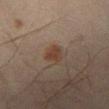biopsy status = no biopsy performed (imaged during a skin exam) | location = the leg | image source = ~15 mm crop, total-body skin-cancer survey | tile lighting = cross-polarized | diameter = ≈2.5 mm | automated lesion analysis = about 6 CIELAB-L* units darker than the surrounding skin and a normalized lesion–skin contrast near 7; a border-irregularity rating of about 3.5/10 and radial color variation of about 0.5; a classifier nevus-likeness of about 95/100 and lesion-presence confidence of about 100/100 | subject = male, about 45 years old.